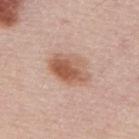Captured during whole-body skin photography for melanoma surveillance; the lesion was not biopsied. A male patient, aged around 60. A region of skin cropped from a whole-body photographic capture, roughly 15 mm wide. On the mid back. Imaged with white-light lighting.